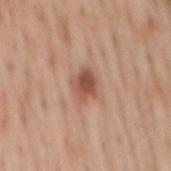| feature | finding |
|---|---|
| notes | total-body-photography surveillance lesion; no biopsy |
| TBP lesion metrics | an average lesion color of about L≈52 a*≈23 b*≈28 (CIELAB); border irregularity of about 2.5 on a 0–10 scale and a peripheral color-asymmetry measure near 1.5 |
| location | the mid back |
| lesion diameter | ≈3 mm |
| patient | male, approximately 60 years of age |
| image | total-body-photography crop, ~15 mm field of view |
| illumination | white-light illumination |